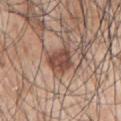Assessment: Imaged during a routine full-body skin examination; the lesion was not biopsied and no histopathology is available. Context: Located on the left arm. This is a white-light tile. A 15 mm close-up tile from a total-body photography series done for melanoma screening. The total-body-photography lesion software estimated an area of roughly 8.5 mm², a shape eccentricity near 0.7, and a shape-asymmetry score of about 0.3 (0 = symmetric). The lesion's longest dimension is about 4 mm. A male subject aged 68–72.